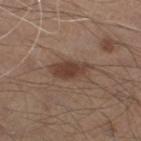Impression:
No biopsy was performed on this lesion — it was imaged during a full skin examination and was not determined to be concerning.
Background:
On the left lower leg. Captured under white-light illumination. The lesion's longest dimension is about 5 mm. A 15 mm close-up extracted from a 3D total-body photography capture. The total-body-photography lesion software estimated an area of roughly 9.5 mm², a shape eccentricity near 0.85, and two-axis asymmetry of about 0.25. It also reported an average lesion color of about L≈41 a*≈17 b*≈25 (CIELAB), roughly 10 lightness units darker than nearby skin, and a normalized border contrast of about 8. The analysis additionally found a border-irregularity index near 3/10 and a color-variation rating of about 3/10. A male patient, approximately 55 years of age.